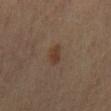Acquisition and patient details: A female patient, in their mid-60s. From the lower back. Imaged with cross-polarized lighting. An algorithmic analysis of the crop reported a shape eccentricity near 0.85 and two-axis asymmetry of about 0.35. It also reported border irregularity of about 3.5 on a 0–10 scale, a within-lesion color-variation index near 1/10, and a peripheral color-asymmetry measure near 0.5. It also reported lesion-presence confidence of about 100/100. A roughly 15 mm field-of-view crop from a total-body skin photograph.